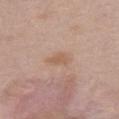Q: Was a biopsy performed?
A: total-body-photography surveillance lesion; no biopsy
Q: Lesion location?
A: the abdomen
Q: How was the tile lit?
A: white-light illumination
Q: What is the imaging modality?
A: ~15 mm crop, total-body skin-cancer survey
Q: Who is the patient?
A: female, aged around 40
Q: How large is the lesion?
A: ≈2.5 mm